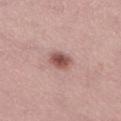Imaged with white-light lighting.
This image is a 15 mm lesion crop taken from a total-body photograph.
A male patient, approximately 55 years of age.
The recorded lesion diameter is about 2.5 mm.
The lesion is on the lower back.